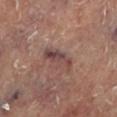Impression:
Imaged during a routine full-body skin examination; the lesion was not biopsied and no histopathology is available.